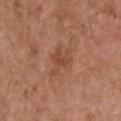notes = imaged on a skin check; not biopsied
image = ~15 mm crop, total-body skin-cancer survey
tile lighting = white-light
subject = male, in their mid-50s
location = the upper back
automated metrics = a lesion color around L≈47 a*≈23 b*≈30 in CIELAB, roughly 7 lightness units darker than nearby skin, and a normalized border contrast of about 5.5